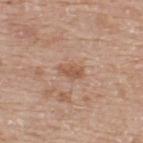Assessment: The lesion was tiled from a total-body skin photograph and was not biopsied. Acquisition and patient details: A male patient, aged 73–77. The tile uses white-light illumination. From the upper back. Longest diameter approximately 3 mm. A 15 mm close-up extracted from a 3D total-body photography capture.